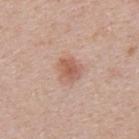follow-up = imaged on a skin check; not biopsied
subject = male, aged approximately 40
lesion diameter = ≈3 mm
illumination = white-light
imaging modality = ~15 mm crop, total-body skin-cancer survey
anatomic site = the upper back